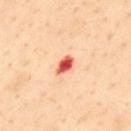notes: total-body-photography surveillance lesion; no biopsy | imaging modality: 15 mm crop, total-body photography | TBP lesion metrics: about 21 CIELAB-L* units darker than the surrounding skin and a lesion-to-skin contrast of about 12 (normalized; higher = more distinct); a classifier nevus-likeness of about 0/100 and lesion-presence confidence of about 100/100 | anatomic site: the upper back | subject: male, aged approximately 55.